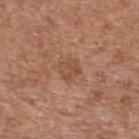Clinical impression: No biopsy was performed on this lesion — it was imaged during a full skin examination and was not determined to be concerning. Context: Cropped from a total-body skin-imaging series; the visible field is about 15 mm. Imaged with white-light lighting. Approximately 3.5 mm at its widest. The lesion is located on the upper back. The patient is a male aged approximately 65. The total-body-photography lesion software estimated an eccentricity of roughly 0.55 and a shape-asymmetry score of about 0.2 (0 = symmetric). The software also gave a mean CIELAB color near L≈51 a*≈21 b*≈30 and roughly 6 lightness units darker than nearby skin. And it measured a classifier nevus-likeness of about 0/100 and lesion-presence confidence of about 100/100.